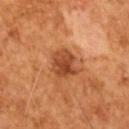Impression: Imaged during a routine full-body skin examination; the lesion was not biopsied and no histopathology is available. Context: This image is a 15 mm lesion crop taken from a total-body photograph. Approximately 3.5 mm at its widest. The tile uses cross-polarized illumination. A male patient, aged 63–67. The total-body-photography lesion software estimated a lesion area of about 8.5 mm², an eccentricity of roughly 0.35, and two-axis asymmetry of about 0.25. The analysis additionally found a mean CIELAB color near L≈46 a*≈28 b*≈38, about 12 CIELAB-L* units darker than the surrounding skin, and a normalized lesion–skin contrast near 9. The analysis additionally found a classifier nevus-likeness of about 40/100 and a lesion-detection confidence of about 100/100.